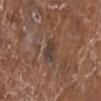Q: Was a biopsy performed?
A: total-body-photography surveillance lesion; no biopsy
Q: What is the anatomic site?
A: the left lower leg
Q: How was this image acquired?
A: ~15 mm crop, total-body skin-cancer survey
Q: What are the patient's age and sex?
A: female, roughly 75 years of age
Q: How was the tile lit?
A: white-light